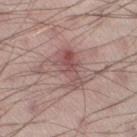Q: Was a biopsy performed?
A: imaged on a skin check; not biopsied
Q: What lighting was used for the tile?
A: white-light illumination
Q: Who is the patient?
A: male, aged around 30
Q: How large is the lesion?
A: ≈7.5 mm
Q: What is the anatomic site?
A: the right thigh
Q: What did automated image analysis measure?
A: an area of roughly 19 mm², a shape eccentricity near 0.75, and a symmetry-axis asymmetry near 0.4; a lesion color around L≈54 a*≈18 b*≈20 in CIELAB and a normalized lesion–skin contrast near 6.5; a border-irregularity index near 7/10, a within-lesion color-variation index near 7.5/10, and radial color variation of about 2; a nevus-likeness score of about 0/100 and lesion-presence confidence of about 100/100
Q: What is the imaging modality?
A: ~15 mm tile from a whole-body skin photo